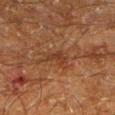No biopsy was performed on this lesion — it was imaged during a full skin examination and was not determined to be concerning. From the left lower leg. The patient is a male aged around 60. Automated tile analysis of the lesion measured a footprint of about 3 mm² and a shape eccentricity near 0.85. The analysis additionally found a border-irregularity index near 4/10, internal color variation of about 0.5 on a 0–10 scale, and peripheral color asymmetry of about 0. The analysis additionally found a nevus-likeness score of about 0/100. A lesion tile, about 15 mm wide, cut from a 3D total-body photograph. About 2.5 mm across. Captured under cross-polarized illumination.